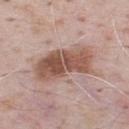Q: Was a biopsy performed?
A: no biopsy performed (imaged during a skin exam)
Q: Automated lesion metrics?
A: a mean CIELAB color near L≈53 a*≈20 b*≈26, a lesion–skin lightness drop of about 13, and a lesion-to-skin contrast of about 9.5 (normalized; higher = more distinct); an automated nevus-likeness rating near 95 out of 100 and a detector confidence of about 100 out of 100 that the crop contains a lesion
Q: What is the anatomic site?
A: the upper back
Q: How was this image acquired?
A: total-body-photography crop, ~15 mm field of view
Q: Illumination type?
A: white-light
Q: What are the patient's age and sex?
A: male, about 70 years old